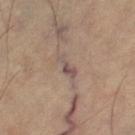  lighting: cross-polarized
  lesion_size:
    long_diameter_mm_approx: 3.5
  automated_metrics:
    vs_skin_darker_L: 9.0
    vs_skin_contrast_norm: 7.5
    nevus_likeness_0_100: 0
  site: right thigh
  image:
    source: total-body photography crop
    field_of_view_mm: 15